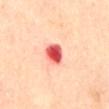Assessment:
Captured during whole-body skin photography for melanoma surveillance; the lesion was not biopsied.
Context:
Cropped from a whole-body photographic skin survey; the tile spans about 15 mm. On the abdomen. A male subject aged 83 or older. The total-body-photography lesion software estimated a lesion area of about 6 mm² and a shape eccentricity near 0.65. It also reported a normalized border contrast of about 12. It also reported a peripheral color-asymmetry measure near 1.5. The analysis additionally found a nevus-likeness score of about 0/100. The recorded lesion diameter is about 3 mm. Imaged with cross-polarized lighting.